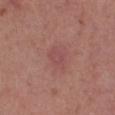This lesion was catalogued during total-body skin photography and was not selected for biopsy. Automated image analysis of the tile measured an automated nevus-likeness rating near 0 out of 100 and lesion-presence confidence of about 100/100. A roughly 15 mm field-of-view crop from a total-body skin photograph. A female patient, aged approximately 40. Imaged with white-light lighting. The lesion is on the left lower leg. About 3 mm across.